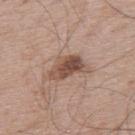workup: no biopsy performed (imaged during a skin exam).